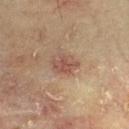notes: catalogued during a skin exam; not biopsied | lighting: cross-polarized illumination | site: the chest | size: ~3.5 mm (longest diameter) | image: ~15 mm tile from a whole-body skin photo | subject: male, aged 68 to 72 | automated metrics: a lesion area of about 5.5 mm², an outline eccentricity of about 0.7 (0 = round, 1 = elongated), and two-axis asymmetry of about 0.3; a lesion color around L≈41 a*≈17 b*≈22 in CIELAB, roughly 8 lightness units darker than nearby skin, and a normalized lesion–skin contrast near 7; a detector confidence of about 100 out of 100 that the crop contains a lesion.